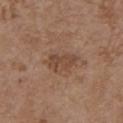workup = catalogued during a skin exam; not biopsied | subject = female, aged 63–67 | lesion diameter = ≈4 mm | site = the chest | acquisition = ~15 mm tile from a whole-body skin photo | image-analysis metrics = a border-irregularity rating of about 4.5/10, a color-variation rating of about 2/10, and a peripheral color-asymmetry measure near 0.5.